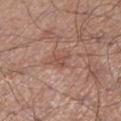acquisition: ~15 mm tile from a whole-body skin photo | illumination: white-light | automated lesion analysis: an area of roughly 3 mm² and an eccentricity of roughly 0.75; border irregularity of about 3.5 on a 0–10 scale and a peripheral color-asymmetry measure near 0.5 | diameter: about 2.5 mm | location: the left lower leg | patient: male, aged around 70.